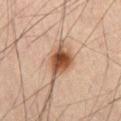Recorded during total-body skin imaging; not selected for excision or biopsy.
Located on the lower back.
A roughly 15 mm field-of-view crop from a total-body skin photograph.
A male patient, in their mid- to late 60s.
The lesion's longest dimension is about 3.5 mm.